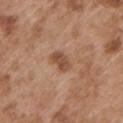No biopsy was performed on this lesion — it was imaged during a full skin examination and was not determined to be concerning. Located on the left upper arm. The subject is a male aged around 55. A close-up tile cropped from a whole-body skin photograph, about 15 mm across. The recorded lesion diameter is about 3 mm. An algorithmic analysis of the crop reported an outline eccentricity of about 0.8 (0 = round, 1 = elongated) and a shape-asymmetry score of about 0.25 (0 = symmetric). The software also gave a mean CIELAB color near L≈49 a*≈21 b*≈31, roughly 10 lightness units darker than nearby skin, and a lesion-to-skin contrast of about 7.5 (normalized; higher = more distinct). It also reported a nevus-likeness score of about 40/100.